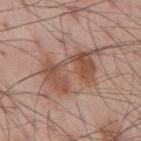The lesion was photographed on a routine skin check and not biopsied; there is no pathology result. On the mid back. A male patient aged 53 to 57. A roughly 15 mm field-of-view crop from a total-body skin photograph. The total-body-photography lesion software estimated a shape eccentricity near 0.8 and two-axis asymmetry of about 0.4. The software also gave roughly 10 lightness units darker than nearby skin and a lesion-to-skin contrast of about 7.5 (normalized; higher = more distinct). The analysis additionally found a border-irregularity index near 7.5/10, internal color variation of about 5.5 on a 0–10 scale, and radial color variation of about 1.5. It also reported a detector confidence of about 100 out of 100 that the crop contains a lesion. The tile uses white-light illumination. Measured at roughly 6.5 mm in maximum diameter.